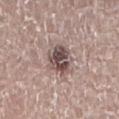Captured under white-light illumination. Located on the lower back. The patient is a male aged approximately 60. Approximately 4 mm at its widest. A 15 mm crop from a total-body photograph taken for skin-cancer surveillance.